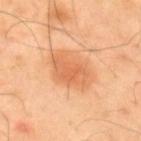This lesion was catalogued during total-body skin photography and was not selected for biopsy. Cropped from a whole-body photographic skin survey; the tile spans about 15 mm. The lesion is on the upper back. This is a cross-polarized tile. A male subject, aged 38 to 42. About 5 mm across. Automated tile analysis of the lesion measured an area of roughly 14 mm² and a shape-asymmetry score of about 0.2 (0 = symmetric). The software also gave internal color variation of about 3.5 on a 0–10 scale and radial color variation of about 1. The analysis additionally found a classifier nevus-likeness of about 95/100 and lesion-presence confidence of about 100/100.